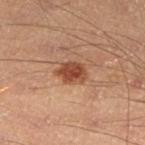<lesion>
  <biopsy_status>not biopsied; imaged during a skin examination</biopsy_status>
  <site>leg</site>
  <patient>
    <sex>male</sex>
    <age_approx>40</age_approx>
  </patient>
  <image>
    <source>total-body photography crop</source>
    <field_of_view_mm>15</field_of_view_mm>
  </image>
</lesion>